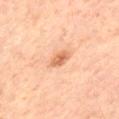Q: Is there a histopathology result?
A: imaged on a skin check; not biopsied
Q: What kind of image is this?
A: ~15 mm tile from a whole-body skin photo
Q: What is the anatomic site?
A: the left thigh
Q: How large is the lesion?
A: ≈2.5 mm
Q: What are the patient's age and sex?
A: male, aged approximately 65